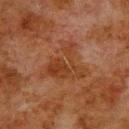Part of a total-body skin-imaging series; this lesion was reviewed on a skin check and was not flagged for biopsy.